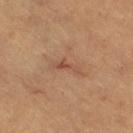The lesion was photographed on a routine skin check and not biopsied; there is no pathology result. The tile uses cross-polarized illumination. A female subject, roughly 45 years of age. Approximately 3.5 mm at its widest. On the leg. Automated tile analysis of the lesion measured a lesion area of about 3 mm², an eccentricity of roughly 0.95, and a shape-asymmetry score of about 0.5 (0 = symmetric). A lesion tile, about 15 mm wide, cut from a 3D total-body photograph.